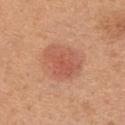On the upper back. A female patient, aged 23 to 27. Imaged with white-light lighting. A close-up tile cropped from a whole-body skin photograph, about 15 mm across. About 5 mm across. The lesion-visualizer software estimated a border-irregularity index near 2/10 and a peripheral color-asymmetry measure near 1.